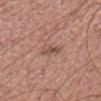follow-up: no biopsy performed (imaged during a skin exam) | acquisition: ~15 mm crop, total-body skin-cancer survey | tile lighting: white-light | patient: male, roughly 50 years of age | location: the head or neck | image-analysis metrics: about 9 CIELAB-L* units darker than the surrounding skin and a normalized lesion–skin contrast near 6.5; a border-irregularity index near 4/10, a color-variation rating of about 0/10, and peripheral color asymmetry of about 0.